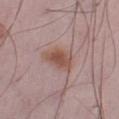Recorded during total-body skin imaging; not selected for excision or biopsy. A male subject roughly 50 years of age. About 4.5 mm across. Cropped from a total-body skin-imaging series; the visible field is about 15 mm. Automated image analysis of the tile measured a nevus-likeness score of about 90/100. On the left lower leg.